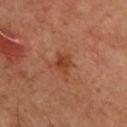Q: Is there a histopathology result?
A: catalogued during a skin exam; not biopsied
Q: Who is the patient?
A: male, in their 50s
Q: Where on the body is the lesion?
A: the chest
Q: How was this image acquired?
A: ~15 mm tile from a whole-body skin photo
Q: What is the lesion's diameter?
A: ≈2.5 mm